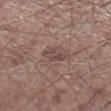notes: imaged on a skin check; not biopsied
automated metrics: a lesion area of about 5 mm², an outline eccentricity of about 0.85 (0 = round, 1 = elongated), and a shape-asymmetry score of about 0.3 (0 = symmetric); a lesion color around L≈46 a*≈17 b*≈20 in CIELAB and a normalized border contrast of about 6; a border-irregularity rating of about 3.5/10, internal color variation of about 3 on a 0–10 scale, and peripheral color asymmetry of about 1; a classifier nevus-likeness of about 0/100 and a lesion-detection confidence of about 100/100
anatomic site: the right lower leg
subject: male, approximately 60 years of age
lighting: white-light
lesion diameter: ~3.5 mm (longest diameter)
acquisition: ~15 mm tile from a whole-body skin photo